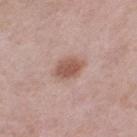| field | value |
|---|---|
| biopsy status | total-body-photography surveillance lesion; no biopsy |
| body site | the left thigh |
| size | about 3.5 mm |
| tile lighting | white-light |
| image | total-body-photography crop, ~15 mm field of view |
| patient | female, about 55 years old |